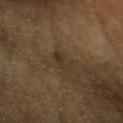Impression: This lesion was catalogued during total-body skin photography and was not selected for biopsy. Image and clinical context: The subject is a male aged 83 or over. Automated tile analysis of the lesion measured an average lesion color of about L≈26 a*≈11 b*≈23 (CIELAB) and a normalized lesion–skin contrast near 6. The software also gave border irregularity of about 5.5 on a 0–10 scale, a color-variation rating of about 0.5/10, and a peripheral color-asymmetry measure near 0. A lesion tile, about 15 mm wide, cut from a 3D total-body photograph. The lesion's longest dimension is about 2.5 mm. The tile uses cross-polarized illumination. Located on the head or neck.